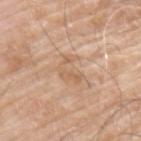A male patient, aged 63 to 67. Located on the back. This image is a 15 mm lesion crop taken from a total-body photograph. Imaged with white-light lighting. About 3 mm across.